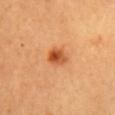Notes:
- follow-up · imaged on a skin check; not biopsied
- imaging modality · ~15 mm crop, total-body skin-cancer survey
- size · ≈3 mm
- patient · female, in their 30s
- anatomic site · the chest